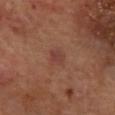No biopsy was performed on this lesion — it was imaged during a full skin examination and was not determined to be concerning.
An algorithmic analysis of the crop reported a normalized lesion–skin contrast near 5.5.
Cropped from a whole-body photographic skin survey; the tile spans about 15 mm.
About 2.5 mm across.
On the mid back.
Captured under cross-polarized illumination.
A male patient, aged 68 to 72.